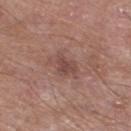follow-up — no biopsy performed (imaged during a skin exam) | acquisition — 15 mm crop, total-body photography | image-analysis metrics — a footprint of about 4.5 mm², a shape eccentricity near 0.55, and a symmetry-axis asymmetry near 0.4; a mean CIELAB color near L≈46 a*≈20 b*≈23, about 8 CIELAB-L* units darker than the surrounding skin, and a lesion-to-skin contrast of about 6.5 (normalized; higher = more distinct); a border-irregularity rating of about 4/10, a within-lesion color-variation index near 1.5/10, and a peripheral color-asymmetry measure near 0.5; an automated nevus-likeness rating near 0 out of 100 | subject — male, roughly 55 years of age | site — the left lower leg | diameter — ≈3 mm | lighting — white-light.